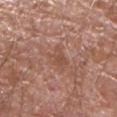Imaged during a routine full-body skin examination; the lesion was not biopsied and no histopathology is available.
On the right upper arm.
A lesion tile, about 15 mm wide, cut from a 3D total-body photograph.
A male subject in their 80s.
This is a white-light tile.